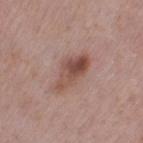Clinical impression: This lesion was catalogued during total-body skin photography and was not selected for biopsy. Background: From the left thigh. About 5.5 mm across. A roughly 15 mm field-of-view crop from a total-body skin photograph. Automated image analysis of the tile measured a color-variation rating of about 8.5/10. Captured under white-light illumination. A female subject, about 40 years old.